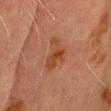Assessment:
The lesion was tiled from a total-body skin photograph and was not biopsied.
Context:
From the head or neck. Automated image analysis of the tile measured a lesion area of about 9.5 mm², an outline eccentricity of about 0.85 (0 = round, 1 = elongated), and two-axis asymmetry of about 0.45. The analysis additionally found a lesion color around L≈38 a*≈21 b*≈31 in CIELAB and a lesion-to-skin contrast of about 7 (normalized; higher = more distinct). And it measured a nevus-likeness score of about 10/100. The subject is a male in their 70s. A region of skin cropped from a whole-body photographic capture, roughly 15 mm wide. The tile uses cross-polarized illumination.